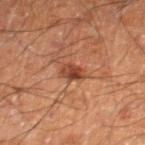follow-up = no biopsy performed (imaged during a skin exam) | location = the leg | illumination = cross-polarized illumination | subject = male, roughly 60 years of age | diameter = ≈3 mm | acquisition = ~15 mm tile from a whole-body skin photo.